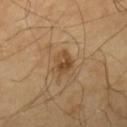<case>
  <biopsy_status>not biopsied; imaged during a skin examination</biopsy_status>
  <automated_metrics>
    <area_mm2_approx>5.0</area_mm2_approx>
    <shape_asymmetry>0.2</shape_asymmetry>
    <nevus_likeness_0_100>55</nevus_likeness_0_100>
    <lesion_detection_confidence_0_100>100</lesion_detection_confidence_0_100>
  </automated_metrics>
  <image>
    <source>total-body photography crop</source>
    <field_of_view_mm>15</field_of_view_mm>
  </image>
  <site>right lower leg</site>
  <lesion_size>
    <long_diameter_mm_approx>2.5</long_diameter_mm_approx>
  </lesion_size>
  <patient>
    <sex>male</sex>
    <age_approx>65</age_approx>
  </patient>
  <lighting>cross-polarized</lighting>
</case>